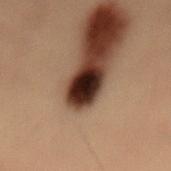Q: Was a biopsy performed?
A: total-body-photography surveillance lesion; no biopsy
Q: Illumination type?
A: cross-polarized
Q: How was this image acquired?
A: ~15 mm crop, total-body skin-cancer survey
Q: What are the patient's age and sex?
A: male, in their mid- to late 50s
Q: How large is the lesion?
A: ~4 mm (longest diameter)
Q: Where on the body is the lesion?
A: the lower back